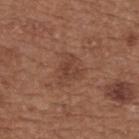{
  "biopsy_status": "not biopsied; imaged during a skin examination",
  "lighting": "white-light",
  "image": {
    "source": "total-body photography crop",
    "field_of_view_mm": 15
  },
  "patient": {
    "sex": "female",
    "age_approx": 65
  },
  "automated_metrics": {
    "area_mm2_approx": 4.0,
    "cielab_L": 41,
    "cielab_a": 21,
    "cielab_b": 27,
    "vs_skin_contrast_norm": 5.5
  },
  "site": "upper back"
}